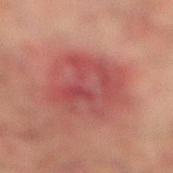Context:
The tile uses cross-polarized illumination. Measured at roughly 8 mm in maximum diameter. The patient is a male aged 73 to 77. An algorithmic analysis of the crop reported a lesion area of about 33 mm² and an eccentricity of roughly 0.65. And it measured a border-irregularity index near 2.5/10 and a within-lesion color-variation index near 6.5/10. The lesion is on the right lower leg. A close-up tile cropped from a whole-body skin photograph, about 15 mm across.